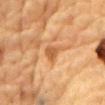Findings:
– follow-up — total-body-photography surveillance lesion; no biopsy
– lesion size — about 2.5 mm
– image — 15 mm crop, total-body photography
– TBP lesion metrics — a lesion area of about 4 mm² and a shape-asymmetry score of about 0.4 (0 = symmetric); a mean CIELAB color near L≈51 a*≈22 b*≈38 and roughly 9 lightness units darker than nearby skin
– anatomic site — the chest
– illumination — cross-polarized
– patient — male, about 85 years old or older